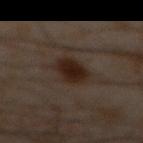Part of a total-body skin-imaging series; this lesion was reviewed on a skin check and was not flagged for biopsy. Measured at roughly 4 mm in maximum diameter. From the front of the torso. A male subject, in their 60s. A region of skin cropped from a whole-body photographic capture, roughly 15 mm wide. The tile uses cross-polarized illumination. Automated image analysis of the tile measured a border-irregularity rating of about 2/10, a within-lesion color-variation index near 3/10, and radial color variation of about 1.